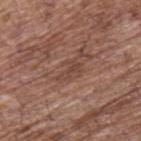{"image": {"source": "total-body photography crop", "field_of_view_mm": 15}, "lighting": "white-light", "lesion_size": {"long_diameter_mm_approx": 3.5}, "patient": {"sex": "male", "age_approx": 70}, "site": "upper back"}